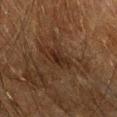tile lighting: cross-polarized | image source: total-body-photography crop, ~15 mm field of view | subject: male, roughly 60 years of age | location: the left forearm | lesion diameter: ≈3 mm.